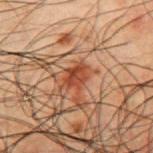Assessment:
Part of a total-body skin-imaging series; this lesion was reviewed on a skin check and was not flagged for biopsy.
Acquisition and patient details:
The lesion is located on the left upper arm. A lesion tile, about 15 mm wide, cut from a 3D total-body photograph. The tile uses cross-polarized illumination. A male subject, in their 50s. The lesion-visualizer software estimated about 9 CIELAB-L* units darker than the surrounding skin. It also reported a classifier nevus-likeness of about 75/100 and a detector confidence of about 100 out of 100 that the crop contains a lesion. Measured at roughly 3 mm in maximum diameter.